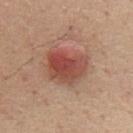notes: imaged on a skin check; not biopsied
acquisition: 15 mm crop, total-body photography
tile lighting: white-light
site: the front of the torso
automated lesion analysis: an area of roughly 16 mm², a shape eccentricity near 0.6, and a shape-asymmetry score of about 0.2 (0 = symmetric); border irregularity of about 2.5 on a 0–10 scale, a color-variation rating of about 8/10, and radial color variation of about 3; a detector confidence of about 100 out of 100 that the crop contains a lesion
subject: female, approximately 50 years of age